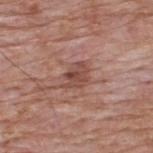Part of a total-body skin-imaging series; this lesion was reviewed on a skin check and was not flagged for biopsy.
A lesion tile, about 15 mm wide, cut from a 3D total-body photograph.
Imaged with white-light lighting.
The lesion is on the upper back.
The subject is a male in their 60s.